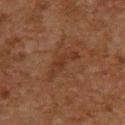The recorded lesion diameter is about 4.5 mm.
A 15 mm close-up extracted from a 3D total-body photography capture.
The subject is a female aged around 60.
The lesion is located on the upper back.
The lesion-visualizer software estimated a footprint of about 6 mm², an outline eccentricity of about 0.95 (0 = round, 1 = elongated), and two-axis asymmetry of about 0.55. And it measured a mean CIELAB color near L≈31 a*≈19 b*≈28, roughly 6 lightness units darker than nearby skin, and a lesion-to-skin contrast of about 6 (normalized; higher = more distinct). And it measured a nevus-likeness score of about 0/100 and a detector confidence of about 100 out of 100 that the crop contains a lesion.
Imaged with cross-polarized lighting.